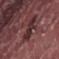Assessment: Recorded during total-body skin imaging; not selected for excision or biopsy. Background: A 15 mm close-up extracted from a 3D total-body photography capture. Measured at roughly 4.5 mm in maximum diameter. A male patient, in their 40s. An algorithmic analysis of the crop reported a mean CIELAB color near L≈31 a*≈22 b*≈17, a lesion–skin lightness drop of about 9, and a normalized lesion–skin contrast near 8. It also reported internal color variation of about 4 on a 0–10 scale. And it measured a nevus-likeness score of about 0/100 and lesion-presence confidence of about 95/100. Imaged with white-light lighting. Located on the abdomen.